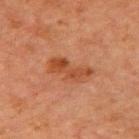The lesion was tiled from a total-body skin photograph and was not biopsied. Cropped from a whole-body photographic skin survey; the tile spans about 15 mm. The lesion is located on the left upper arm. A male patient, aged 63–67. An algorithmic analysis of the crop reported an outline eccentricity of about 0.9 (0 = round, 1 = elongated) and a symmetry-axis asymmetry near 0.45. The software also gave a mean CIELAB color near L≈38 a*≈23 b*≈31, a lesion–skin lightness drop of about 8, and a normalized lesion–skin contrast near 7.5.